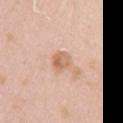Clinical impression:
The lesion was tiled from a total-body skin photograph and was not biopsied.
Image and clinical context:
The lesion-visualizer software estimated roughly 10 lightness units darker than nearby skin and a normalized lesion–skin contrast near 7. This is a white-light tile. The subject is a female about 40 years old. A lesion tile, about 15 mm wide, cut from a 3D total-body photograph. The lesion is located on the left arm.